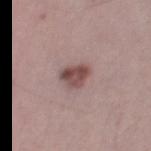The lesion was tiled from a total-body skin photograph and was not biopsied. Located on the right thigh. A male subject, aged 43–47. Cropped from a total-body skin-imaging series; the visible field is about 15 mm. Approximately 3 mm at its widest.